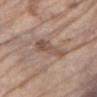| feature | finding |
|---|---|
| workup | total-body-photography surveillance lesion; no biopsy |
| image source | 15 mm crop, total-body photography |
| lesion size | ~4 mm (longest diameter) |
| lighting | white-light |
| TBP lesion metrics | an average lesion color of about L≈52 a*≈18 b*≈25 (CIELAB) and a normalized lesion–skin contrast near 7; a classifier nevus-likeness of about 40/100 and a detector confidence of about 100 out of 100 that the crop contains a lesion |
| subject | female, in their 70s |
| location | the right forearm |